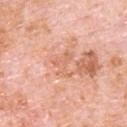workup: catalogued during a skin exam; not biopsied | automated lesion analysis: an area of roughly 4.5 mm² and a shape-asymmetry score of about 0.75 (0 = symmetric); about 7 CIELAB-L* units darker than the surrounding skin and a normalized lesion–skin contrast near 4.5 | tile lighting: white-light illumination | acquisition: ~15 mm crop, total-body skin-cancer survey | subject: male, aged 78 to 82 | anatomic site: the back | diameter: about 3 mm.